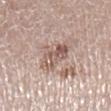The lesion was tiled from a total-body skin photograph and was not biopsied. A region of skin cropped from a whole-body photographic capture, roughly 15 mm wide. This is a white-light tile. Automated image analysis of the tile measured a lesion area of about 7 mm², a shape eccentricity near 0.8, and a symmetry-axis asymmetry near 0.2. The software also gave lesion-presence confidence of about 100/100. Approximately 4 mm at its widest. The subject is a female in their 50s. The lesion is on the right lower leg.